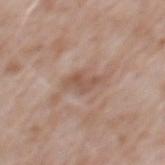illumination = white-light; patient = male, aged approximately 50; automated metrics = an eccentricity of roughly 0.85 and two-axis asymmetry of about 0.4; body site = the upper back; diameter = ~3.5 mm (longest diameter); acquisition = ~15 mm tile from a whole-body skin photo.